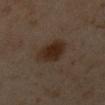Assessment:
No biopsy was performed on this lesion — it was imaged during a full skin examination and was not determined to be concerning.
Context:
Automated tile analysis of the lesion measured a lesion area of about 10 mm², an eccentricity of roughly 0.45, and two-axis asymmetry of about 0.15. A 15 mm close-up tile from a total-body photography series done for melanoma screening. A male patient in their 60s. Located on the chest. Imaged with cross-polarized lighting.